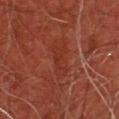workup: imaged on a skin check; not biopsied | imaging modality: 15 mm crop, total-body photography | location: the upper back | subject: male, aged 43 to 47.